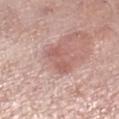This lesion was catalogued during total-body skin photography and was not selected for biopsy. A region of skin cropped from a whole-body photographic capture, roughly 15 mm wide. On the right lower leg. Captured under white-light illumination. A female patient roughly 70 years of age.